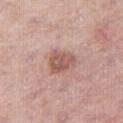biopsy status = catalogued during a skin exam; not biopsied
illumination = white-light
image = 15 mm crop, total-body photography
subject = male, aged approximately 75
automated lesion analysis = a lesion color around L≈56 a*≈22 b*≈25 in CIELAB, roughly 11 lightness units darker than nearby skin, and a normalized lesion–skin contrast near 7.5; a border-irregularity index near 2.5/10, internal color variation of about 3 on a 0–10 scale, and peripheral color asymmetry of about 1; a detector confidence of about 100 out of 100 that the crop contains a lesion
location = the right thigh